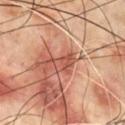Case summary:
• biopsy status · no biopsy performed (imaged during a skin exam)
• lesion diameter · ~3 mm (longest diameter)
• subject · male, aged 68 to 72
• imaging modality · ~15 mm crop, total-body skin-cancer survey
• site · the chest
• lighting · cross-polarized
• automated lesion analysis · an area of roughly 4 mm², an eccentricity of roughly 0.75, and a symmetry-axis asymmetry near 0.45; an average lesion color of about L≈50 a*≈27 b*≈30 (CIELAB), about 9 CIELAB-L* units darker than the surrounding skin, and a normalized lesion–skin contrast near 6; an automated nevus-likeness rating near 0 out of 100 and lesion-presence confidence of about 95/100